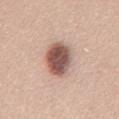Q: Is there a histopathology result?
A: catalogued during a skin exam; not biopsied
Q: Where on the body is the lesion?
A: the front of the torso
Q: How was the tile lit?
A: white-light
Q: What kind of image is this?
A: 15 mm crop, total-body photography
Q: What are the patient's age and sex?
A: male, aged 43 to 47
Q: What did automated image analysis measure?
A: an average lesion color of about L≈55 a*≈20 b*≈24 (CIELAB), roughly 18 lightness units darker than nearby skin, and a normalized border contrast of about 11.5
Q: How large is the lesion?
A: about 5 mm